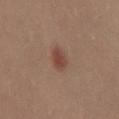Assessment: Part of a total-body skin-imaging series; this lesion was reviewed on a skin check and was not flagged for biopsy. Acquisition and patient details: Imaged with cross-polarized lighting. Located on the left leg. A female subject aged around 40. A region of skin cropped from a whole-body photographic capture, roughly 15 mm wide. Approximately 3.5 mm at its widest. The total-body-photography lesion software estimated a lesion area of about 4.5 mm² and an outline eccentricity of about 0.9 (0 = round, 1 = elongated). The software also gave a mean CIELAB color near L≈44 a*≈21 b*≈26, roughly 9 lightness units darker than nearby skin, and a normalized border contrast of about 7.5. The software also gave a border-irregularity rating of about 2.5/10, internal color variation of about 2 on a 0–10 scale, and a peripheral color-asymmetry measure near 1.